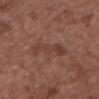This lesion was catalogued during total-body skin photography and was not selected for biopsy. An algorithmic analysis of the crop reported a lesion–skin lightness drop of about 6 and a normalized lesion–skin contrast near 5. And it measured a border-irregularity index near 5/10 and internal color variation of about 3 on a 0–10 scale. The analysis additionally found a detector confidence of about 100 out of 100 that the crop contains a lesion. Cropped from a total-body skin-imaging series; the visible field is about 15 mm. The patient is a male aged approximately 50. Measured at roughly 5.5 mm in maximum diameter. Located on the head or neck. Imaged with white-light lighting.